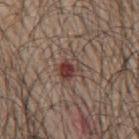biopsy status = no biopsy performed (imaged during a skin exam)
anatomic site = the mid back
tile lighting = white-light illumination
size = ≈2.5 mm
image = ~15 mm tile from a whole-body skin photo
patient = male, roughly 70 years of age